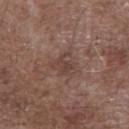Q: Was a biopsy performed?
A: catalogued during a skin exam; not biopsied
Q: Where on the body is the lesion?
A: the front of the torso
Q: Lesion size?
A: ≈3 mm
Q: How was the tile lit?
A: white-light
Q: Who is the patient?
A: male, aged 68–72
Q: What kind of image is this?
A: ~15 mm tile from a whole-body skin photo